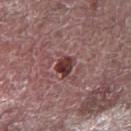Clinical impression: Recorded during total-body skin imaging; not selected for excision or biopsy. Image and clinical context: A 15 mm close-up tile from a total-body photography series done for melanoma screening. On the right forearm. A male patient approximately 65 years of age. About 2.5 mm across. The tile uses white-light illumination.